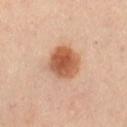Assessment:
The lesion was photographed on a routine skin check and not biopsied; there is no pathology result.
Background:
A female subject, aged 38 to 42. On the right leg. A close-up tile cropped from a whole-body skin photograph, about 15 mm across. Automated tile analysis of the lesion measured a footprint of about 12 mm², a shape eccentricity near 0.2, and two-axis asymmetry of about 0.1. It also reported a border-irregularity index near 1/10, internal color variation of about 5 on a 0–10 scale, and a peripheral color-asymmetry measure near 1.5. This is a cross-polarized tile.